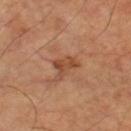<record>
  <biopsy_status>not biopsied; imaged during a skin examination</biopsy_status>
</record>